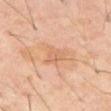• biopsy status — imaged on a skin check; not biopsied
• anatomic site — the abdomen
• tile lighting — cross-polarized
• subject — male, roughly 60 years of age
• image source — ~15 mm crop, total-body skin-cancer survey